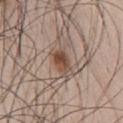Part of a total-body skin-imaging series; this lesion was reviewed on a skin check and was not flagged for biopsy. About 4 mm across. A male patient about 35 years old. Automated tile analysis of the lesion measured a footprint of about 7 mm², an eccentricity of roughly 0.85, and two-axis asymmetry of about 0.25. The analysis additionally found a lesion color around L≈49 a*≈17 b*≈26 in CIELAB, a lesion–skin lightness drop of about 11, and a normalized lesion–skin contrast near 8. The analysis additionally found a color-variation rating of about 6.5/10. Located on the chest. Captured under white-light illumination. A close-up tile cropped from a whole-body skin photograph, about 15 mm across.